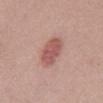The lesion was photographed on a routine skin check and not biopsied; there is no pathology result. The lesion is located on the mid back. The subject is a female aged approximately 40. Measured at roughly 4 mm in maximum diameter. A 15 mm close-up tile from a total-body photography series done for melanoma screening. Captured under white-light illumination.